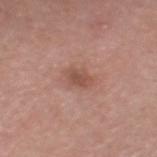The lesion was photographed on a routine skin check and not biopsied; there is no pathology result. This is a white-light tile. A 15 mm close-up tile from a total-body photography series done for melanoma screening. The lesion is located on the head or neck. About 3 mm across. The patient is a male about 50 years old.